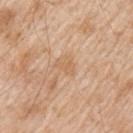<tbp_lesion>
  <biopsy_status>not biopsied; imaged during a skin examination</biopsy_status>
</tbp_lesion>